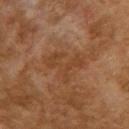illumination = cross-polarized illumination
diameter = about 5 mm
imaging modality = ~15 mm crop, total-body skin-cancer survey
anatomic site = the back
subject = female, in their 60s